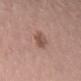Impression:
Part of a total-body skin-imaging series; this lesion was reviewed on a skin check and was not flagged for biopsy.
Clinical summary:
On the left thigh. The recorded lesion diameter is about 3.5 mm. A lesion tile, about 15 mm wide, cut from a 3D total-body photograph. A female patient, aged around 25.